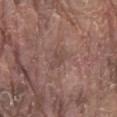  biopsy_status: not biopsied; imaged during a skin examination
  automated_metrics:
    cielab_L: 46
    cielab_a: 18
    cielab_b: 21
    vs_skin_darker_L: 6.0
    vs_skin_contrast_norm: 4.5
    border_irregularity_0_10: 3.0
    color_variation_0_10: 2.0
    peripheral_color_asymmetry: 0.5
  image:
    source: total-body photography crop
    field_of_view_mm: 15
  patient:
    sex: male
    age_approx: 80
  site: abdomen